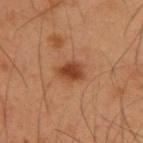Context: A male subject, approximately 55 years of age. From the upper back. A close-up tile cropped from a whole-body skin photograph, about 15 mm across.